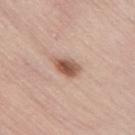<lesion>
  <biopsy_status>not biopsied; imaged during a skin examination</biopsy_status>
  <lesion_size>
    <long_diameter_mm_approx>3.0</long_diameter_mm_approx>
  </lesion_size>
  <image>
    <source>total-body photography crop</source>
    <field_of_view_mm>15</field_of_view_mm>
  </image>
  <site>right upper arm</site>
  <lighting>white-light</lighting>
  <automated_metrics>
    <area_mm2_approx>5.5</area_mm2_approx>
    <eccentricity>0.7</eccentricity>
    <shape_asymmetry>0.25</shape_asymmetry>
  </automated_metrics>
  <patient>
    <sex>female</sex>
    <age_approx>45</age_approx>
  </patient>
</lesion>